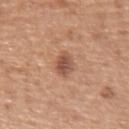Clinical impression:
The lesion was tiled from a total-body skin photograph and was not biopsied.
Context:
A 15 mm crop from a total-body photograph taken for skin-cancer surveillance. Captured under white-light illumination. A male patient, aged approximately 55. The lesion is on the right upper arm. About 3 mm across. An algorithmic analysis of the crop reported an area of roughly 5 mm², an outline eccentricity of about 0.7 (0 = round, 1 = elongated), and a symmetry-axis asymmetry near 0.2. The analysis additionally found a lesion color around L≈52 a*≈22 b*≈29 in CIELAB. The software also gave a nevus-likeness score of about 65/100 and a lesion-detection confidence of about 100/100.